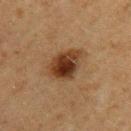  biopsy_status: not biopsied; imaged during a skin examination
  site: left upper arm
  image:
    source: total-body photography crop
    field_of_view_mm: 15
  lighting: cross-polarized
  lesion_size:
    long_diameter_mm_approx: 5.0
  patient:
    sex: female
    age_approx: 60